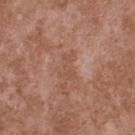workup = catalogued during a skin exam; not biopsied | image-analysis metrics = an automated nevus-likeness rating near 0 out of 100 and lesion-presence confidence of about 100/100 | site = the upper back | lighting = white-light | patient = male, in their mid- to late 40s | acquisition = ~15 mm tile from a whole-body skin photo | diameter = ~3 mm (longest diameter).